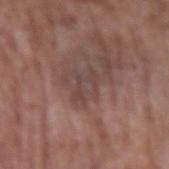Acquisition and patient details:
Measured at roughly 3 mm in maximum diameter. On the left upper arm. An algorithmic analysis of the crop reported a nevus-likeness score of about 0/100 and a lesion-detection confidence of about 90/100. Imaged with white-light lighting. A male subject approximately 65 years of age. A 15 mm close-up tile from a total-body photography series done for melanoma screening.